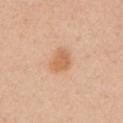biopsy_status: not biopsied; imaged during a skin examination
patient:
  sex: female
  age_approx: 40
lesion_size:
  long_diameter_mm_approx: 3.0
image:
  source: total-body photography crop
  field_of_view_mm: 15
site: left upper arm
automated_metrics:
  area_mm2_approx: 6.0
  eccentricity: 0.6
  shape_asymmetry: 0.3
  nevus_likeness_0_100: 90
  lesion_detection_confidence_0_100: 100